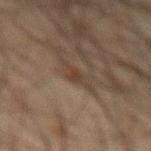Clinical impression:
This lesion was catalogued during total-body skin photography and was not selected for biopsy.
Context:
Longest diameter approximately 3.5 mm. The lesion is located on the abdomen. An algorithmic analysis of the crop reported a footprint of about 3.5 mm², a shape eccentricity near 0.9, and two-axis asymmetry of about 0.5. The software also gave a border-irregularity rating of about 5.5/10 and a color-variation rating of about 1.5/10. A male subject about 65 years old. Captured under cross-polarized illumination. A 15 mm close-up extracted from a 3D total-body photography capture.